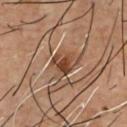{
  "biopsy_status": "not biopsied; imaged during a skin examination",
  "lighting": "cross-polarized",
  "automated_metrics": {
    "cielab_L": 41,
    "cielab_a": 21,
    "cielab_b": 30,
    "vs_skin_contrast_norm": 9.5,
    "nevus_likeness_0_100": 50,
    "lesion_detection_confidence_0_100": 95
  },
  "patient": {
    "sex": "male",
    "age_approx": 55
  },
  "site": "chest",
  "image": {
    "source": "total-body photography crop",
    "field_of_view_mm": 15
  },
  "lesion_size": {
    "long_diameter_mm_approx": 2.5
  }
}